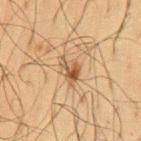No biopsy was performed on this lesion — it was imaged during a full skin examination and was not determined to be concerning. This is a cross-polarized tile. The total-body-photography lesion software estimated an area of roughly 5 mm², an eccentricity of roughly 0.8, and a shape-asymmetry score of about 0.35 (0 = symmetric). The analysis additionally found a lesion color around L≈43 a*≈15 b*≈29 in CIELAB and a lesion-to-skin contrast of about 7.5 (normalized; higher = more distinct). The analysis additionally found a border-irregularity index near 3.5/10, a within-lesion color-variation index near 6.5/10, and a peripheral color-asymmetry measure near 2. Cropped from a total-body skin-imaging series; the visible field is about 15 mm. The subject is a male aged 58–62. On the chest. The lesion's longest dimension is about 3.5 mm.